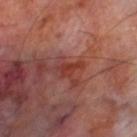size = about 2.5 mm | tile lighting = cross-polarized illumination | subject = male, aged 68–72 | TBP lesion metrics = an automated nevus-likeness rating near 0 out of 100 | location = the left thigh | imaging modality = 15 mm crop, total-body photography.